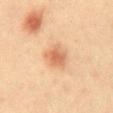A male subject aged 38 to 42. A roughly 15 mm field-of-view crop from a total-body skin photograph. This is a cross-polarized tile. Located on the front of the torso.